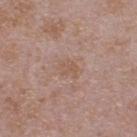Q: Is there a histopathology result?
A: total-body-photography surveillance lesion; no biopsy
Q: How large is the lesion?
A: ≈2.5 mm
Q: Automated lesion metrics?
A: an eccentricity of roughly 0.65 and two-axis asymmetry of about 0.25; about 5 CIELAB-L* units darker than the surrounding skin and a normalized lesion–skin contrast near 5; a classifier nevus-likeness of about 0/100 and a detector confidence of about 100 out of 100 that the crop contains a lesion
Q: What are the patient's age and sex?
A: female, aged around 25
Q: What kind of image is this?
A: total-body-photography crop, ~15 mm field of view
Q: Where on the body is the lesion?
A: the head or neck